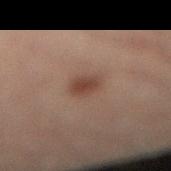biopsy status = catalogued during a skin exam; not biopsied
lighting = cross-polarized
subject = male, aged 28–32
lesion diameter = ~3 mm (longest diameter)
anatomic site = the right lower leg
automated metrics = an area of roughly 5 mm², an eccentricity of roughly 0.55, and two-axis asymmetry of about 0.15
image = 15 mm crop, total-body photography